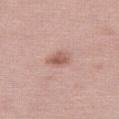Part of a total-body skin-imaging series; this lesion was reviewed on a skin check and was not flagged for biopsy.
The tile uses white-light illumination.
The lesion's longest dimension is about 2.5 mm.
A female patient aged 38–42.
Automated tile analysis of the lesion measured a footprint of about 4.5 mm² and a shape eccentricity near 0.7. It also reported an average lesion color of about L≈58 a*≈22 b*≈26 (CIELAB), about 11 CIELAB-L* units darker than the surrounding skin, and a lesion-to-skin contrast of about 7 (normalized; higher = more distinct). The analysis additionally found a border-irregularity index near 2/10, internal color variation of about 4 on a 0–10 scale, and radial color variation of about 1.5. It also reported a nevus-likeness score of about 55/100.
The lesion is on the right thigh.
A roughly 15 mm field-of-view crop from a total-body skin photograph.